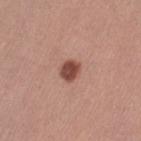<case>
  <biopsy_status>not biopsied; imaged during a skin examination</biopsy_status>
  <site>left thigh</site>
  <image>
    <source>total-body photography crop</source>
    <field_of_view_mm>15</field_of_view_mm>
  </image>
  <patient>
    <sex>female</sex>
    <age_approx>45</age_approx>
  </patient>
</case>